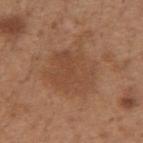Recorded during total-body skin imaging; not selected for excision or biopsy. A 15 mm close-up extracted from a 3D total-body photography capture. The lesion is on the left forearm. A female subject about 30 years old.